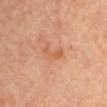Recorded during total-body skin imaging; not selected for excision or biopsy.